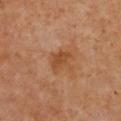biopsy status: catalogued during a skin exam; not biopsied | lesion size: about 3 mm | body site: the chest | patient: female, aged 53–57 | automated metrics: a lesion color around L≈49 a*≈24 b*≈38 in CIELAB, a lesion–skin lightness drop of about 8, and a normalized border contrast of about 6.5 | acquisition: 15 mm crop, total-body photography | illumination: cross-polarized.